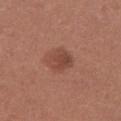<lesion>
  <biopsy_status>not biopsied; imaged during a skin examination</biopsy_status>
  <site>chest</site>
  <image>
    <source>total-body photography crop</source>
    <field_of_view_mm>15</field_of_view_mm>
  </image>
  <patient>
    <sex>female</sex>
    <age_approx>25</age_approx>
  </patient>
  <automated_metrics>
    <area_mm2_approx>6.5</area_mm2_approx>
    <eccentricity>0.4</eccentricity>
    <shape_asymmetry>0.25</shape_asymmetry>
    <border_irregularity_0_10>2.0</border_irregularity_0_10>
    <peripheral_color_asymmetry>1.0</peripheral_color_asymmetry>
  </automated_metrics>
  <lesion_size>
    <long_diameter_mm_approx>3.0</long_diameter_mm_approx>
  </lesion_size>
</lesion>